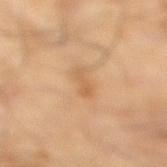workup: catalogued during a skin exam; not biopsied
patient: male, aged 63 to 67
illumination: cross-polarized
diameter: about 2.5 mm
image source: ~15 mm tile from a whole-body skin photo
image-analysis metrics: a lesion–skin lightness drop of about 7; a classifier nevus-likeness of about 0/100 and a lesion-detection confidence of about 100/100
anatomic site: the right lower leg